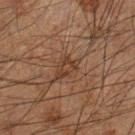<record>
  <biopsy_status>not biopsied; imaged during a skin examination</biopsy_status>
  <site>left forearm</site>
  <lighting>cross-polarized</lighting>
  <patient>
    <sex>male</sex>
    <age_approx>65</age_approx>
  </patient>
  <image>
    <source>total-body photography crop</source>
    <field_of_view_mm>15</field_of_view_mm>
  </image>
  <lesion_size>
    <long_diameter_mm_approx>3.5</long_diameter_mm_approx>
  </lesion_size>
</record>